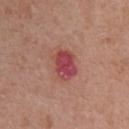<record>
  <biopsy_status>not biopsied; imaged during a skin examination</biopsy_status>
  <site>upper back</site>
  <patient>
    <sex>female</sex>
    <age_approx>40</age_approx>
  </patient>
  <automated_metrics>
    <cielab_L>46</cielab_L>
    <cielab_a>33</cielab_a>
    <cielab_b>22</cielab_b>
    <vs_skin_contrast_norm>9.0</vs_skin_contrast_norm>
  </automated_metrics>
  <lesion_size>
    <long_diameter_mm_approx>3.5</long_diameter_mm_approx>
  </lesion_size>
  <lighting>white-light</lighting>
  <image>
    <source>total-body photography crop</source>
    <field_of_view_mm>15</field_of_view_mm>
  </image>
</record>